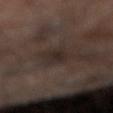Clinical impression: Recorded during total-body skin imaging; not selected for excision or biopsy. Context: A close-up tile cropped from a whole-body skin photograph, about 15 mm across. The lesion is on the right lower leg.